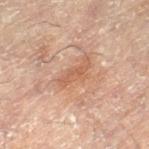A close-up tile cropped from a whole-body skin photograph, about 15 mm across. The lesion is on the right leg. Approximately 3.5 mm at its widest. A female patient in their 80s.